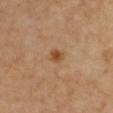Captured during whole-body skin photography for melanoma surveillance; the lesion was not biopsied. A lesion tile, about 15 mm wide, cut from a 3D total-body photograph. Automated tile analysis of the lesion measured a mean CIELAB color near L≈50 a*≈20 b*≈37 and roughly 10 lightness units darker than nearby skin. And it measured a detector confidence of about 100 out of 100 that the crop contains a lesion. A female subject aged approximately 50. Imaged with cross-polarized lighting. Approximately 2 mm at its widest. Located on the chest.